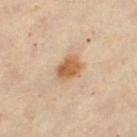This lesion was catalogued during total-body skin photography and was not selected for biopsy.
Longest diameter approximately 3.5 mm.
The tile uses cross-polarized illumination.
A 15 mm close-up tile from a total-body photography series done for melanoma screening.
A female patient, in their 60s.
The lesion is located on the left thigh.